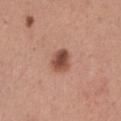The lesion was tiled from a total-body skin photograph and was not biopsied.
The recorded lesion diameter is about 2.5 mm.
Located on the left thigh.
The patient is a female aged around 30.
A 15 mm close-up extracted from a 3D total-body photography capture.
The tile uses white-light illumination.
The lesion-visualizer software estimated an area of roughly 5.5 mm² and a symmetry-axis asymmetry near 0.15. It also reported a mean CIELAB color near L≈49 a*≈24 b*≈28 and about 14 CIELAB-L* units darker than the surrounding skin. And it measured an automated nevus-likeness rating near 95 out of 100 and lesion-presence confidence of about 100/100.